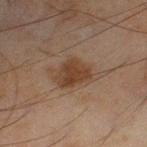{"biopsy_status": "not biopsied; imaged during a skin examination", "lesion_size": {"long_diameter_mm_approx": 5.5}, "site": "left lower leg", "image": {"source": "total-body photography crop", "field_of_view_mm": 15}, "patient": {"sex": "male", "age_approx": 45}}